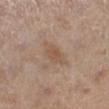The lesion was tiled from a total-body skin photograph and was not biopsied. From the left lower leg. Measured at roughly 4 mm in maximum diameter. Cropped from a whole-body photographic skin survey; the tile spans about 15 mm. This is a white-light tile. A female patient about 40 years old.